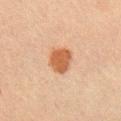Case summary:
- workup — imaged on a skin check; not biopsied
- anatomic site — the right upper arm
- imaging modality — total-body-photography crop, ~15 mm field of view
- patient — male, about 60 years old